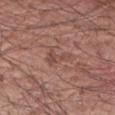biopsy status: no biopsy performed (imaged during a skin exam) | image: ~15 mm crop, total-body skin-cancer survey | patient: male, aged approximately 60 | body site: the arm.